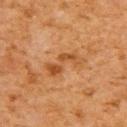| field | value |
|---|---|
| notes | catalogued during a skin exam; not biopsied |
| location | the upper back |
| automated metrics | a lesion–skin lightness drop of about 8 and a lesion-to-skin contrast of about 6.5 (normalized; higher = more distinct); a border-irregularity rating of about 6.5/10 and a peripheral color-asymmetry measure near 1; a nevus-likeness score of about 0/100 and lesion-presence confidence of about 100/100 |
| size | about 5 mm |
| tile lighting | cross-polarized |
| imaging modality | 15 mm crop, total-body photography |
| patient | male, about 60 years old |